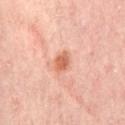Q: What kind of image is this?
A: ~15 mm tile from a whole-body skin photo
Q: What did automated image analysis measure?
A: an eccentricity of roughly 0.7 and a symmetry-axis asymmetry near 0.2; border irregularity of about 1.5 on a 0–10 scale and internal color variation of about 2.5 on a 0–10 scale
Q: What is the anatomic site?
A: the lower back
Q: How large is the lesion?
A: about 2.5 mm
Q: What lighting was used for the tile?
A: cross-polarized illumination
Q: Who is the patient?
A: in their mid-50s